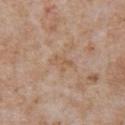The lesion was photographed on a routine skin check and not biopsied; there is no pathology result. Measured at roughly 3 mm in maximum diameter. From the chest. This image is a 15 mm lesion crop taken from a total-body photograph. Captured under white-light illumination. A male patient, roughly 65 years of age. Automated tile analysis of the lesion measured a lesion area of about 3.5 mm², an outline eccentricity of about 0.85 (0 = round, 1 = elongated), and a shape-asymmetry score of about 0.35 (0 = symmetric). The software also gave a lesion color around L≈57 a*≈17 b*≈32 in CIELAB, a lesion–skin lightness drop of about 6, and a lesion-to-skin contrast of about 5 (normalized; higher = more distinct). It also reported a lesion-detection confidence of about 100/100.